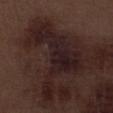Part of a total-body skin-imaging series; this lesion was reviewed on a skin check and was not flagged for biopsy. The lesion is on the right lower leg. A male patient, aged 68–72. The lesion's longest dimension is about 11 mm. Automated tile analysis of the lesion measured a border-irregularity rating of about 10/10, a within-lesion color-variation index near 3.5/10, and radial color variation of about 1. This is a white-light tile. A 15 mm close-up tile from a total-body photography series done for melanoma screening.